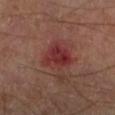Q: Is there a histopathology result?
A: no biopsy performed (imaged during a skin exam)
Q: How was this image acquired?
A: 15 mm crop, total-body photography
Q: Who is the patient?
A: male, roughly 70 years of age
Q: Where on the body is the lesion?
A: the left lower leg
Q: What did automated image analysis measure?
A: a shape-asymmetry score of about 0.3 (0 = symmetric); a lesion–skin lightness drop of about 8 and a lesion-to-skin contrast of about 8 (normalized; higher = more distinct); border irregularity of about 2.5 on a 0–10 scale, internal color variation of about 4.5 on a 0–10 scale, and radial color variation of about 1.5